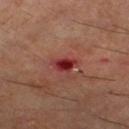Clinical impression:
No biopsy was performed on this lesion — it was imaged during a full skin examination and was not determined to be concerning.
Image and clinical context:
Located on the leg. Cropped from a total-body skin-imaging series; the visible field is about 15 mm. The patient is a male aged around 60. The tile uses cross-polarized illumination.